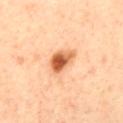Imaged during a routine full-body skin examination; the lesion was not biopsied and no histopathology is available.
A region of skin cropped from a whole-body photographic capture, roughly 15 mm wide.
The lesion is on the mid back.
A male patient, in their 40s.